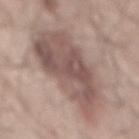The lesion was tiled from a total-body skin photograph and was not biopsied. The patient is a male approximately 55 years of age. A roughly 15 mm field-of-view crop from a total-body skin photograph. Located on the mid back.